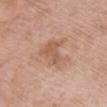{"biopsy_status": "not biopsied; imaged during a skin examination", "site": "chest", "patient": {"sex": "female", "age_approx": 70}, "image": {"source": "total-body photography crop", "field_of_view_mm": 15}}